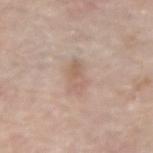<record>
<biopsy_status>not biopsied; imaged during a skin examination</biopsy_status>
<site>right forearm</site>
<image>
  <source>total-body photography crop</source>
  <field_of_view_mm>15</field_of_view_mm>
</image>
<lesion_size>
  <long_diameter_mm_approx>4.0</long_diameter_mm_approx>
</lesion_size>
<patient>
  <sex>female</sex>
  <age_approx>70</age_approx>
</patient>
<automated_metrics>
  <eccentricity>0.85</eccentricity>
  <shape_asymmetry>0.25</shape_asymmetry>
</automated_metrics>
</record>